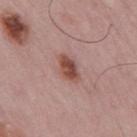Part of a total-body skin-imaging series; this lesion was reviewed on a skin check and was not flagged for biopsy. The subject is a male aged 48–52. Measured at roughly 3.5 mm in maximum diameter. The total-body-photography lesion software estimated a border-irregularity rating of about 2.5/10, a color-variation rating of about 3.5/10, and peripheral color asymmetry of about 1. Located on the mid back. A 15 mm close-up tile from a total-body photography series done for melanoma screening.